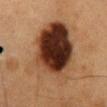<tbp_lesion>
  <patient>
    <sex>male</sex>
    <age_approx>55</age_approx>
  </patient>
  <site>mid back</site>
  <image>
    <source>total-body photography crop</source>
    <field_of_view_mm>15</field_of_view_mm>
  </image>
</tbp_lesion>